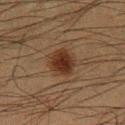The lesion was photographed on a routine skin check and not biopsied; there is no pathology result. The subject is a male aged 33–37. An algorithmic analysis of the crop reported an eccentricity of roughly 0.3 and a symmetry-axis asymmetry near 0.15. It also reported a lesion color around L≈25 a*≈16 b*≈23 in CIELAB, a lesion–skin lightness drop of about 9, and a normalized border contrast of about 10. The analysis additionally found border irregularity of about 2 on a 0–10 scale, a color-variation rating of about 3/10, and radial color variation of about 1. The tile uses cross-polarized illumination. A region of skin cropped from a whole-body photographic capture, roughly 15 mm wide. On the leg.